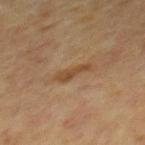This lesion was catalogued during total-body skin photography and was not selected for biopsy.
A region of skin cropped from a whole-body photographic capture, roughly 15 mm wide.
The recorded lesion diameter is about 3.5 mm.
The lesion-visualizer software estimated a footprint of about 4 mm² and a shape eccentricity near 0.95. The software also gave a lesion–skin lightness drop of about 7 and a lesion-to-skin contrast of about 7 (normalized; higher = more distinct). And it measured a nevus-likeness score of about 0/100 and a lesion-detection confidence of about 100/100.
The lesion is on the mid back.
Captured under cross-polarized illumination.
The patient is a male aged approximately 65.